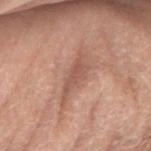Q: Is there a histopathology result?
A: catalogued during a skin exam; not biopsied
Q: What is the lesion's diameter?
A: about 4 mm
Q: How was the tile lit?
A: cross-polarized illumination
Q: Patient demographics?
A: male, aged approximately 70
Q: What is the anatomic site?
A: the right forearm
Q: How was this image acquired?
A: ~15 mm crop, total-body skin-cancer survey